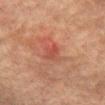Part of a total-body skin-imaging series; this lesion was reviewed on a skin check and was not flagged for biopsy. A male patient, aged approximately 75. Cropped from a whole-body photographic skin survey; the tile spans about 15 mm. Automated tile analysis of the lesion measured a footprint of about 3 mm², a shape eccentricity near 0.8, and a symmetry-axis asymmetry near 0.25. The software also gave a lesion color around L≈41 a*≈26 b*≈27 in CIELAB and a lesion–skin lightness drop of about 6. The software also gave a border-irregularity rating of about 2.5/10 and peripheral color asymmetry of about 0.5. And it measured a detector confidence of about 100 out of 100 that the crop contains a lesion. From the abdomen. Imaged with cross-polarized lighting. Longest diameter approximately 2.5 mm.